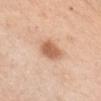| key | value |
|---|---|
| follow-up | total-body-photography surveillance lesion; no biopsy |
| site | the front of the torso |
| imaging modality | 15 mm crop, total-body photography |
| subject | female, about 65 years old |